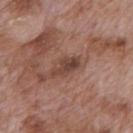{
  "biopsy_status": "not biopsied; imaged during a skin examination",
  "image": {
    "source": "total-body photography crop",
    "field_of_view_mm": 15
  },
  "patient": {
    "sex": "male",
    "age_approx": 70
  },
  "lighting": "white-light",
  "site": "back",
  "lesion_size": {
    "long_diameter_mm_approx": 3.5
  }
}